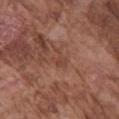The lesion was photographed on a routine skin check and not biopsied; there is no pathology result. A 15 mm crop from a total-body photograph taken for skin-cancer surveillance. This is a white-light tile. A male subject, in their mid-70s. The recorded lesion diameter is about 2.5 mm. The lesion is located on the right upper arm.